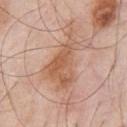Part of a total-body skin-imaging series; this lesion was reviewed on a skin check and was not flagged for biopsy.
A 15 mm crop from a total-body photograph taken for skin-cancer surveillance.
The tile uses white-light illumination.
The lesion is on the chest.
The lesion-visualizer software estimated a footprint of about 19 mm², an eccentricity of roughly 0.85, and a symmetry-axis asymmetry near 0.45. The software also gave a border-irregularity rating of about 7/10 and a within-lesion color-variation index near 4.5/10.
A male patient, aged around 55.